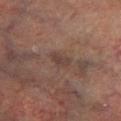biopsy status=no biopsy performed (imaged during a skin exam); acquisition=~15 mm tile from a whole-body skin photo; diameter=~2.5 mm (longest diameter); site=the left lower leg; subject=male, in their mid- to late 70s.